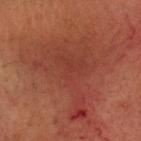{"biopsy_status": "not biopsied; imaged during a skin examination", "lighting": "cross-polarized", "lesion_size": {"long_diameter_mm_approx": 10.0}, "site": "head or neck", "patient": {"sex": "male", "age_approx": 50}, "image": {"source": "total-body photography crop", "field_of_view_mm": 15}, "automated_metrics": {"area_mm2_approx": 30.0, "eccentricity": 0.85, "shape_asymmetry": 0.7, "vs_skin_contrast_norm": 5.0, "nevus_likeness_0_100": 0}}